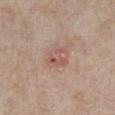follow-up = no biopsy performed (imaged during a skin exam).